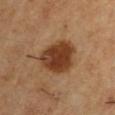Clinical impression: No biopsy was performed on this lesion — it was imaged during a full skin examination and was not determined to be concerning.